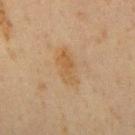Clinical impression: The lesion was photographed on a routine skin check and not biopsied; there is no pathology result. Background: Automated tile analysis of the lesion measured an average lesion color of about L≈46 a*≈15 b*≈33 (CIELAB), a lesion–skin lightness drop of about 6, and a normalized lesion–skin contrast near 6.5. And it measured peripheral color asymmetry of about 1. From the mid back. A lesion tile, about 15 mm wide, cut from a 3D total-body photograph. Approximately 4.5 mm at its widest. The subject is a male approximately 60 years of age.